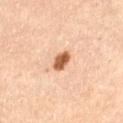No biopsy was performed on this lesion — it was imaged during a full skin examination and was not determined to be concerning.
Automated tile analysis of the lesion measured a lesion area of about 4.5 mm² and a shape-asymmetry score of about 0.2 (0 = symmetric). The analysis additionally found an automated nevus-likeness rating near 100 out of 100 and a lesion-detection confidence of about 100/100.
A roughly 15 mm field-of-view crop from a total-body skin photograph.
A female patient approximately 45 years of age.
From the right thigh.